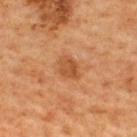Q: Was a biopsy performed?
A: imaged on a skin check; not biopsied
Q: Illumination type?
A: cross-polarized illumination
Q: What is the imaging modality?
A: ~15 mm crop, total-body skin-cancer survey
Q: Lesion size?
A: ≈3 mm
Q: Lesion location?
A: the upper back
Q: What are the patient's age and sex?
A: male, aged around 65